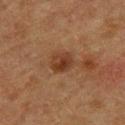The lesion was tiled from a total-body skin photograph and was not biopsied. The lesion is located on the upper back. Imaged with cross-polarized lighting. A female subject, aged 53 to 57. The recorded lesion diameter is about 3 mm. A 15 mm close-up tile from a total-body photography series done for melanoma screening.